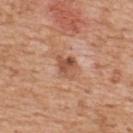The lesion was tiled from a total-body skin photograph and was not biopsied. A male patient, approximately 60 years of age. On the upper back. A close-up tile cropped from a whole-body skin photograph, about 15 mm across.